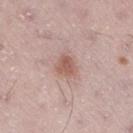Clinical summary: A male patient, in their mid-50s. Located on the right thigh. The tile uses white-light illumination. A 15 mm close-up tile from a total-body photography series done for melanoma screening.